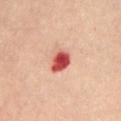Q: Is there a histopathology result?
A: catalogued during a skin exam; not biopsied
Q: How was this image acquired?
A: ~15 mm tile from a whole-body skin photo
Q: Automated lesion metrics?
A: a lesion area of about 6 mm², an eccentricity of roughly 0.65, and a shape-asymmetry score of about 0.25 (0 = symmetric); a lesion color around L≈54 a*≈37 b*≈30 in CIELAB, a lesion–skin lightness drop of about 19, and a normalized border contrast of about 12
Q: Who is the patient?
A: female, aged 43 to 47
Q: What is the anatomic site?
A: the front of the torso
Q: How large is the lesion?
A: ≈3 mm
Q: Illumination type?
A: cross-polarized